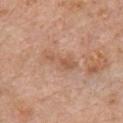Part of a total-body skin-imaging series; this lesion was reviewed on a skin check and was not flagged for biopsy.
Approximately 4.5 mm at its widest.
Captured under white-light illumination.
The lesion-visualizer software estimated a lesion area of about 6.5 mm², an eccentricity of roughly 0.9, and a shape-asymmetry score of about 0.35 (0 = symmetric). The software also gave a lesion color around L≈57 a*≈20 b*≈32 in CIELAB, roughly 7 lightness units darker than nearby skin, and a normalized border contrast of about 5.5. And it measured a border-irregularity index near 4.5/10, a within-lesion color-variation index near 2/10, and a peripheral color-asymmetry measure near 0.5.
A 15 mm crop from a total-body photograph taken for skin-cancer surveillance.
From the chest.
A male patient roughly 60 years of age.